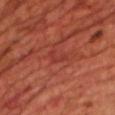workup: no biopsy performed (imaged during a skin exam)
location: the chest
tile lighting: cross-polarized
imaging modality: total-body-photography crop, ~15 mm field of view
subject: male, in their 70s
diameter: ~2.5 mm (longest diameter)